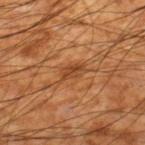The lesion was tiled from a total-body skin photograph and was not biopsied. On the leg. The tile uses cross-polarized illumination. A close-up tile cropped from a whole-body skin photograph, about 15 mm across. The patient is a male aged around 60. Automated tile analysis of the lesion measured a footprint of about 4.5 mm², an outline eccentricity of about 0.85 (0 = round, 1 = elongated), and a symmetry-axis asymmetry near 0.35. The analysis additionally found a within-lesion color-variation index near 3.5/10 and radial color variation of about 1. The analysis additionally found a nevus-likeness score of about 40/100 and a lesion-detection confidence of about 90/100.